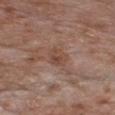Impression:
This lesion was catalogued during total-body skin photography and was not selected for biopsy.
Background:
The lesion is on the chest. Automated tile analysis of the lesion measured a lesion color around L≈46 a*≈20 b*≈26 in CIELAB, a lesion–skin lightness drop of about 7, and a lesion-to-skin contrast of about 6 (normalized; higher = more distinct). The software also gave a classifier nevus-likeness of about 0/100 and a lesion-detection confidence of about 100/100. About 2.5 mm across. A female patient, about 75 years old. A close-up tile cropped from a whole-body skin photograph, about 15 mm across.